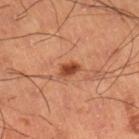Q: Was a biopsy performed?
A: imaged on a skin check; not biopsied
Q: Lesion location?
A: the right lower leg
Q: What kind of image is this?
A: ~15 mm crop, total-body skin-cancer survey
Q: What did automated image analysis measure?
A: a footprint of about 3.5 mm²
Q: Patient demographics?
A: male, aged approximately 60
Q: Lesion size?
A: ≈2.5 mm